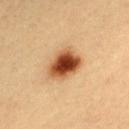The lesion was photographed on a routine skin check and not biopsied; there is no pathology result. From the upper back. Cropped from a whole-body photographic skin survey; the tile spans about 15 mm. The tile uses cross-polarized illumination. A male patient approximately 20 years of age. The lesion-visualizer software estimated a lesion area of about 12 mm², an outline eccentricity of about 0.7 (0 = round, 1 = elongated), and two-axis asymmetry of about 0.25. And it measured an average lesion color of about L≈49 a*≈24 b*≈38 (CIELAB) and a normalized border contrast of about 13. It also reported a border-irregularity index near 2/10, internal color variation of about 8.5 on a 0–10 scale, and peripheral color asymmetry of about 2.5. And it measured lesion-presence confidence of about 100/100.